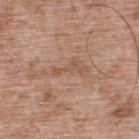Recorded during total-body skin imaging; not selected for excision or biopsy.
The lesion's longest dimension is about 4 mm.
This image is a 15 mm lesion crop taken from a total-body photograph.
Imaged with white-light lighting.
The lesion is on the upper back.
A male subject, in their 50s.